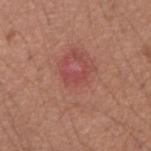Imaged during a routine full-body skin examination; the lesion was not biopsied and no histopathology is available.
Approximately 3 mm at its widest.
The subject is a male aged around 35.
This is a white-light tile.
A region of skin cropped from a whole-body photographic capture, roughly 15 mm wide.
From the arm.